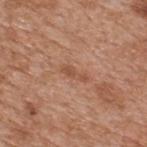Assessment: The lesion was photographed on a routine skin check and not biopsied; there is no pathology result. Background: Located on the upper back. Captured under white-light illumination. The lesion's longest dimension is about 3 mm. A male patient, approximately 65 years of age. A 15 mm close-up extracted from a 3D total-body photography capture.